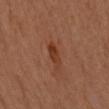biopsy status: no biopsy performed (imaged during a skin exam) | automated metrics: an eccentricity of roughly 0.75 and a symmetry-axis asymmetry near 0.3; a border-irregularity rating of about 3/10 and a peripheral color-asymmetry measure near 1.5 | location: the arm | acquisition: ~15 mm tile from a whole-body skin photo | patient: female, about 60 years old | lesion size: ~3 mm (longest diameter).